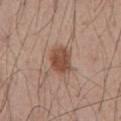biopsy status: no biopsy performed (imaged during a skin exam) | image source: ~15 mm tile from a whole-body skin photo | site: the abdomen | patient: male, approximately 60 years of age | automated metrics: a mean CIELAB color near L≈48 a*≈21 b*≈29 and a lesion-to-skin contrast of about 9 (normalized; higher = more distinct); a border-irregularity index near 1.5/10 and radial color variation of about 1 | lesion size: about 3.5 mm.